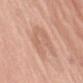notes — no biopsy performed (imaged during a skin exam) | site — the left upper arm | lesion diameter — about 5.5 mm | imaging modality — 15 mm crop, total-body photography | automated lesion analysis — an area of roughly 14 mm², a shape eccentricity near 0.8, and a symmetry-axis asymmetry near 0.35; a lesion color around L≈63 a*≈21 b*≈30 in CIELAB, a lesion–skin lightness drop of about 7, and a normalized border contrast of about 4.5 | lighting — white-light illumination | patient — female, aged 63–67.